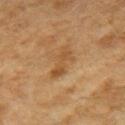The lesion was tiled from a total-body skin photograph and was not biopsied. About 4 mm across. A lesion tile, about 15 mm wide, cut from a 3D total-body photograph. The lesion is located on the arm. An algorithmic analysis of the crop reported a lesion area of about 6 mm², a shape eccentricity near 0.85, and two-axis asymmetry of about 0.3. And it measured an automated nevus-likeness rating near 10 out of 100. A female patient, in their 60s. Imaged with cross-polarized lighting.